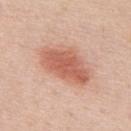notes — no biopsy performed (imaged during a skin exam)
subject — male, aged 58–62
lighting — white-light
site — the mid back
image-analysis metrics — an area of roughly 18 mm² and a shape-asymmetry score of about 0.2 (0 = symmetric); a detector confidence of about 100 out of 100 that the crop contains a lesion
diameter — ≈6.5 mm
image source — ~15 mm crop, total-body skin-cancer survey